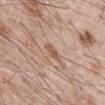{
  "biopsy_status": "not biopsied; imaged during a skin examination",
  "patient": {
    "sex": "male",
    "age_approx": 70
  },
  "image": {
    "source": "total-body photography crop",
    "field_of_view_mm": 15
  },
  "lesion_size": {
    "long_diameter_mm_approx": 3.0
  },
  "automated_metrics": {
    "area_mm2_approx": 3.5,
    "eccentricity": 0.85,
    "shape_asymmetry": 0.35,
    "cielab_L": 57,
    "cielab_a": 19,
    "cielab_b": 29,
    "vs_skin_darker_L": 8.0,
    "vs_skin_contrast_norm": 6.5,
    "border_irregularity_0_10": 3.5,
    "color_variation_0_10": 2.0,
    "peripheral_color_asymmetry": 0.5,
    "nevus_likeness_0_100": 0,
    "lesion_detection_confidence_0_100": 100
  },
  "site": "abdomen",
  "lighting": "white-light"
}